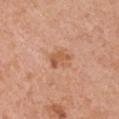follow-up — no biopsy performed (imaged during a skin exam); location — the right upper arm; image-analysis metrics — a lesion area of about 4 mm², an outline eccentricity of about 0.75 (0 = round, 1 = elongated), and a shape-asymmetry score of about 0.25 (0 = symmetric); size — ~3 mm (longest diameter); patient — male, aged 68–72; image — 15 mm crop, total-body photography.